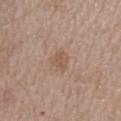| feature | finding |
|---|---|
| biopsy status | imaged on a skin check; not biopsied |
| patient | female, aged 43–47 |
| body site | the upper back |
| lesion diameter | ≈2.5 mm |
| imaging modality | ~15 mm tile from a whole-body skin photo |
| automated metrics | a shape eccentricity near 0.65 and a shape-asymmetry score of about 0.3 (0 = symmetric); a border-irregularity rating of about 3/10, a within-lesion color-variation index near 1.5/10, and peripheral color asymmetry of about 0.5; a nevus-likeness score of about 5/100 and lesion-presence confidence of about 100/100 |